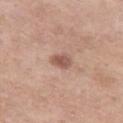Imaged during a routine full-body skin examination; the lesion was not biopsied and no histopathology is available. The lesion-visualizer software estimated a footprint of about 4.5 mm² and a shape-asymmetry score of about 0.25 (0 = symmetric). The analysis additionally found an average lesion color of about L≈55 a*≈21 b*≈26 (CIELAB) and a lesion-to-skin contrast of about 7.5 (normalized; higher = more distinct). And it measured border irregularity of about 2.5 on a 0–10 scale, internal color variation of about 2 on a 0–10 scale, and a peripheral color-asymmetry measure near 0.5. Imaged with white-light lighting. The lesion's longest dimension is about 2.5 mm. A close-up tile cropped from a whole-body skin photograph, about 15 mm across. The lesion is on the leg. A female patient aged around 55.